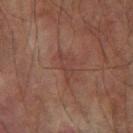| key | value |
|---|---|
| follow-up | no biopsy performed (imaged during a skin exam) |
| patient | male, aged approximately 75 |
| location | the arm |
| lesion size | ≈3.5 mm |
| acquisition | total-body-photography crop, ~15 mm field of view |